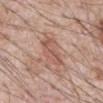workup — no biopsy performed (imaged during a skin exam)
body site — the abdomen
imaging modality — 15 mm crop, total-body photography
lesion size — ~4 mm (longest diameter)
patient — male, in their 70s
illumination — white-light illumination
image-analysis metrics — a lesion area of about 6.5 mm² and a shape-asymmetry score of about 0.35 (0 = symmetric); a mean CIELAB color near L≈55 a*≈22 b*≈27, roughly 8 lightness units darker than nearby skin, and a normalized lesion–skin contrast near 6; a within-lesion color-variation index near 3/10 and a peripheral color-asymmetry measure near 1; lesion-presence confidence of about 100/100